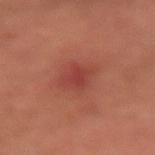Impression: Captured during whole-body skin photography for melanoma surveillance; the lesion was not biopsied. Image and clinical context: The subject is a male aged around 45. On the arm. This image is a 15 mm lesion crop taken from a total-body photograph. Approximately 2.5 mm at its widest. Automated tile analysis of the lesion measured an area of roughly 4.5 mm², an eccentricity of roughly 0.65, and a symmetry-axis asymmetry near 0.3. The software also gave roughly 7 lightness units darker than nearby skin. It also reported internal color variation of about 1.5 on a 0–10 scale and peripheral color asymmetry of about 0.5. The analysis additionally found a classifier nevus-likeness of about 35/100 and a lesion-detection confidence of about 100/100.